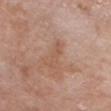Case summary:
• follow-up · imaged on a skin check; not biopsied
• image · total-body-photography crop, ~15 mm field of view
• location · the chest
• illumination · white-light
• patient · female, about 75 years old
• diameter · about 4 mm